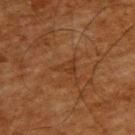Notes:
* notes · imaged on a skin check; not biopsied
* location · the upper back
* automated metrics · an eccentricity of roughly 0.8; a border-irregularity rating of about 5.5/10 and a color-variation rating of about 1.5/10; an automated nevus-likeness rating near 0 out of 100 and a lesion-detection confidence of about 95/100
* image source · ~15 mm tile from a whole-body skin photo
* patient · male, approximately 65 years of age
* size · about 3 mm